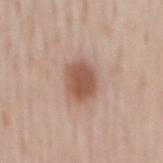{"biopsy_status": "not biopsied; imaged during a skin examination", "patient": {"sex": "male", "age_approx": 65}, "lesion_size": {"long_diameter_mm_approx": 4.0}, "image": {"source": "total-body photography crop", "field_of_view_mm": 15}, "site": "mid back"}